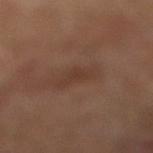Impression: The lesion was tiled from a total-body skin photograph and was not biopsied. Clinical summary: Automated tile analysis of the lesion measured an eccentricity of roughly 0.85. The software also gave a mean CIELAB color near L≈35 a*≈18 b*≈25, roughly 5 lightness units darker than nearby skin, and a lesion-to-skin contrast of about 5 (normalized; higher = more distinct). Captured under cross-polarized illumination. About 3.5 mm across. The lesion is located on the leg. A lesion tile, about 15 mm wide, cut from a 3D total-body photograph. A male subject, aged 63 to 67.